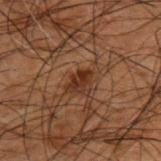Part of a total-body skin-imaging series; this lesion was reviewed on a skin check and was not flagged for biopsy. The patient is a male about 50 years old. A 15 mm crop from a total-body photograph taken for skin-cancer surveillance. The lesion is on the upper back.